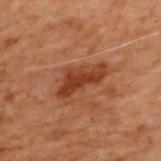{"biopsy_status": "not biopsied; imaged during a skin examination", "lesion_size": {"long_diameter_mm_approx": 5.5}, "site": "upper back", "image": {"source": "total-body photography crop", "field_of_view_mm": 15}, "patient": {"sex": "male", "age_approx": 70}, "lighting": "cross-polarized", "automated_metrics": {"area_mm2_approx": 11.0, "eccentricity": 0.85, "shape_asymmetry": 0.4, "cielab_L": 30, "cielab_a": 21, "cielab_b": 27, "vs_skin_darker_L": 8.0, "peripheral_color_asymmetry": 1.0, "nevus_likeness_0_100": 20, "lesion_detection_confidence_0_100": 100}}